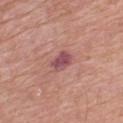Impression:
Part of a total-body skin-imaging series; this lesion was reviewed on a skin check and was not flagged for biopsy.
Acquisition and patient details:
The total-body-photography lesion software estimated a lesion area of about 5 mm², a shape eccentricity near 0.7, and two-axis asymmetry of about 0.2. And it measured a mean CIELAB color near L≈50 a*≈26 b*≈19, a lesion–skin lightness drop of about 11, and a normalized lesion–skin contrast near 9. And it measured a lesion-detection confidence of about 100/100. Captured under white-light illumination. A roughly 15 mm field-of-view crop from a total-body skin photograph. The subject is a male aged approximately 60. The lesion is located on the upper back. The recorded lesion diameter is about 3 mm.